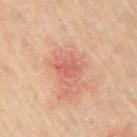Q: Was this lesion biopsied?
A: catalogued during a skin exam; not biopsied
Q: How large is the lesion?
A: ~3 mm (longest diameter)
Q: What is the imaging modality?
A: total-body-photography crop, ~15 mm field of view
Q: Patient demographics?
A: male, aged 58–62
Q: Lesion location?
A: the right upper arm
Q: How was the tile lit?
A: cross-polarized